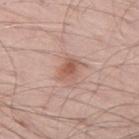* image — ~15 mm crop, total-body skin-cancer survey
* lighting — white-light
* patient — male, approximately 60 years of age
* anatomic site — the left thigh
* diameter — about 3 mm